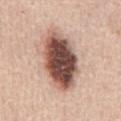biopsy_status: not biopsied; imaged during a skin examination
site: abdomen
lesion_size:
  long_diameter_mm_approx: 8.5
image:
  source: total-body photography crop
  field_of_view_mm: 15
lighting: white-light
patient:
  sex: male
  age_approx: 65